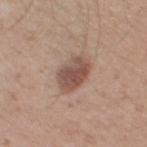follow-up — no biopsy performed (imaged during a skin exam) | acquisition — ~15 mm tile from a whole-body skin photo | patient — male, aged around 65 | site — the leg.